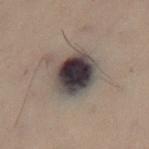Assessment:
This lesion was catalogued during total-body skin photography and was not selected for biopsy.
Clinical summary:
A 15 mm close-up extracted from a 3D total-body photography capture. The total-body-photography lesion software estimated a lesion area of about 17 mm², an eccentricity of roughly 0.45, and a symmetry-axis asymmetry near 0.1. From the right thigh. The subject is a female approximately 50 years of age.